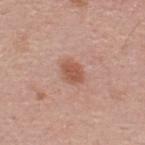Impression:
The lesion was tiled from a total-body skin photograph and was not biopsied.
Image and clinical context:
The subject is a male aged around 45. About 3 mm across. This is a white-light tile. On the upper back. A roughly 15 mm field-of-view crop from a total-body skin photograph.